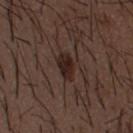Assessment: The lesion was tiled from a total-body skin photograph and was not biopsied. Background: The lesion is on the mid back. A male patient, approximately 50 years of age. Measured at roughly 3.5 mm in maximum diameter. An algorithmic analysis of the crop reported an area of roughly 5.5 mm² and a symmetry-axis asymmetry near 0.2. A 15 mm crop from a total-body photograph taken for skin-cancer surveillance. This is a white-light tile.